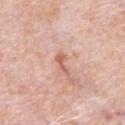Q: Was this lesion biopsied?
A: catalogued during a skin exam; not biopsied
Q: Lesion location?
A: the chest
Q: What are the patient's age and sex?
A: male, in their 80s
Q: How was this image acquired?
A: 15 mm crop, total-body photography
Q: What is the lesion's diameter?
A: ~2.5 mm (longest diameter)
Q: Automated lesion metrics?
A: a footprint of about 2.5 mm², a shape eccentricity near 0.9, and a symmetry-axis asymmetry near 0.5; a border-irregularity rating of about 5/10, a within-lesion color-variation index near 0/10, and radial color variation of about 0